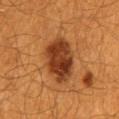{
  "biopsy_status": "not biopsied; imaged during a skin examination",
  "site": "mid back",
  "image": {
    "source": "total-body photography crop",
    "field_of_view_mm": 15
  },
  "patient": {
    "sex": "male",
    "age_approx": 60
  },
  "lesion_size": {
    "long_diameter_mm_approx": 6.0
  },
  "automated_metrics": {
    "area_mm2_approx": 19.0,
    "shape_asymmetry": 0.15
  },
  "lighting": "cross-polarized"
}